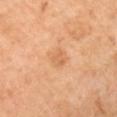Assessment: No biopsy was performed on this lesion — it was imaged during a full skin examination and was not determined to be concerning. Context: The patient is a female roughly 50 years of age. Imaged with cross-polarized lighting. The lesion is located on the right lower leg. This image is a 15 mm lesion crop taken from a total-body photograph.